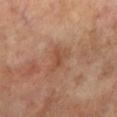Assessment:
This lesion was catalogued during total-body skin photography and was not selected for biopsy.
Image and clinical context:
This is a cross-polarized tile. A male subject in their 70s. A close-up tile cropped from a whole-body skin photograph, about 15 mm across. On the left lower leg.